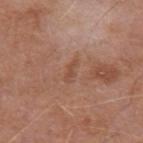No biopsy was performed on this lesion — it was imaged during a full skin examination and was not determined to be concerning. A lesion tile, about 15 mm wide, cut from a 3D total-body photograph. The subject is a male about 65 years old. An algorithmic analysis of the crop reported an area of roughly 2 mm² and a symmetry-axis asymmetry near 0.5. The analysis additionally found a border-irregularity index near 5.5/10, a color-variation rating of about 0/10, and peripheral color asymmetry of about 0. The software also gave lesion-presence confidence of about 100/100. Captured under white-light illumination. Located on the left upper arm.